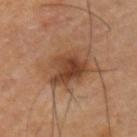<tbp_lesion>
<biopsy_status>not biopsied; imaged during a skin examination</biopsy_status>
<lesion_size>
  <long_diameter_mm_approx>4.5</long_diameter_mm_approx>
</lesion_size>
<image>
  <source>total-body photography crop</source>
  <field_of_view_mm>15</field_of_view_mm>
</image>
<site>left upper arm</site>
<patient>
  <sex>female</sex>
  <age_approx>55</age_approx>
</patient>
<automated_metrics>
  <area_mm2_approx>13.0</area_mm2_approx>
  <eccentricity>0.25</eccentricity>
  <shape_asymmetry>0.25</shape_asymmetry>
  <cielab_L>40</cielab_L>
  <cielab_a>20</cielab_a>
  <cielab_b>29</cielab_b>
  <vs_skin_darker_L>10.0</vs_skin_darker_L>
  <vs_skin_contrast_norm>8.5</vs_skin_contrast_norm>
  <nevus_likeness_0_100>70</nevus_likeness_0_100>
  <lesion_detection_confidence_0_100>100</lesion_detection_confidence_0_100>
</automated_metrics>
</tbp_lesion>